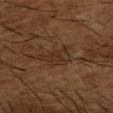follow-up: imaged on a skin check; not biopsied | image source: ~15 mm tile from a whole-body skin photo | body site: the right forearm | subject: male, aged 63 to 67.